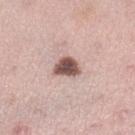Case summary:
• follow-up — no biopsy performed (imaged during a skin exam)
• anatomic site — the left lower leg
• subject — female, aged around 35
• acquisition — total-body-photography crop, ~15 mm field of view